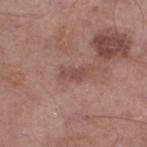notes=catalogued during a skin exam; not biopsied
imaging modality=~15 mm crop, total-body skin-cancer survey
location=the left lower leg
lesion size=~3 mm (longest diameter)
patient=male, roughly 55 years of age